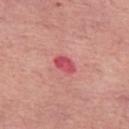{"biopsy_status": "not biopsied; imaged during a skin examination", "patient": {"sex": "female", "age_approx": 55}, "image": {"source": "total-body photography crop", "field_of_view_mm": 15}, "lighting": "white-light", "automated_metrics": {"vs_skin_darker_L": 12.0, "vs_skin_contrast_norm": 8.0, "border_irregularity_0_10": 1.5, "color_variation_0_10": 2.5, "peripheral_color_asymmetry": 1.0, "nevus_likeness_0_100": 0, "lesion_detection_confidence_0_100": 100}, "site": "left thigh", "lesion_size": {"long_diameter_mm_approx": 3.0}}